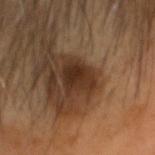{"biopsy_status": "not biopsied; imaged during a skin examination", "lighting": "cross-polarized", "patient": {"sex": "male", "age_approx": 55}, "site": "head or neck", "lesion_size": {"long_diameter_mm_approx": 3.5}, "automated_metrics": {"area_mm2_approx": 8.5, "eccentricity": 0.4, "cielab_L": 27, "cielab_a": 16, "cielab_b": 25, "vs_skin_contrast_norm": 10.0, "nevus_likeness_0_100": 25, "lesion_detection_confidence_0_100": 95}, "image": {"source": "total-body photography crop", "field_of_view_mm": 15}}